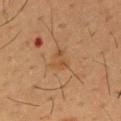{
  "biopsy_status": "not biopsied; imaged during a skin examination",
  "image": {
    "source": "total-body photography crop",
    "field_of_view_mm": 15
  },
  "patient": {
    "sex": "male",
    "age_approx": 55
  },
  "automated_metrics": {
    "cielab_L": 43,
    "cielab_a": 18,
    "cielab_b": 32,
    "vs_skin_darker_L": 5.0,
    "vs_skin_contrast_norm": 5.0,
    "border_irregularity_0_10": 5.0,
    "color_variation_0_10": 1.0,
    "peripheral_color_asymmetry": 0.5,
    "lesion_detection_confidence_0_100": 100
  },
  "site": "chest"
}